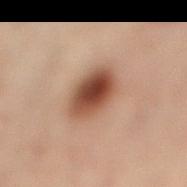| field | value |
|---|---|
| biopsy status | total-body-photography surveillance lesion; no biopsy |
| location | the left leg |
| lesion size | about 4.5 mm |
| patient | female, approximately 55 years of age |
| image source | 15 mm crop, total-body photography |
| automated metrics | border irregularity of about 1 on a 0–10 scale, a within-lesion color-variation index near 6/10, and peripheral color asymmetry of about 1.5 |
| illumination | cross-polarized illumination |